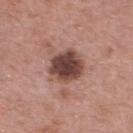| field | value |
|---|---|
| biopsy status | total-body-photography surveillance lesion; no biopsy |
| body site | the upper back |
| acquisition | ~15 mm crop, total-body skin-cancer survey |
| subject | male, aged approximately 65 |